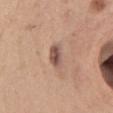Impression: No biopsy was performed on this lesion — it was imaged during a full skin examination and was not determined to be concerning. Background: The subject is a female about 30 years old. Located on the front of the torso. This is a white-light tile. A 15 mm crop from a total-body photograph taken for skin-cancer surveillance.